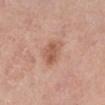biopsy status = imaged on a skin check; not biopsied
illumination = white-light
patient = female, about 65 years old
diameter = ~3.5 mm (longest diameter)
image-analysis metrics = border irregularity of about 2.5 on a 0–10 scale and internal color variation of about 3 on a 0–10 scale; a nevus-likeness score of about 60/100 and lesion-presence confidence of about 100/100
imaging modality = 15 mm crop, total-body photography
site = the right lower leg